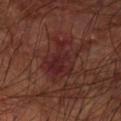This lesion was catalogued during total-body skin photography and was not selected for biopsy.